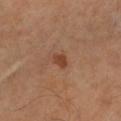Imaged during a routine full-body skin examination; the lesion was not biopsied and no histopathology is available. The lesion's longest dimension is about 2 mm. A lesion tile, about 15 mm wide, cut from a 3D total-body photograph. Automated tile analysis of the lesion measured an average lesion color of about L≈40 a*≈23 b*≈31 (CIELAB), about 9 CIELAB-L* units darker than the surrounding skin, and a normalized lesion–skin contrast near 8. The software also gave a nevus-likeness score of about 80/100 and a detector confidence of about 100 out of 100 that the crop contains a lesion. A male subject aged around 65. The lesion is on the arm. Captured under cross-polarized illumination.